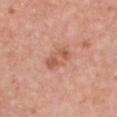Impression: The lesion was tiled from a total-body skin photograph and was not biopsied. Image and clinical context: The recorded lesion diameter is about 3.5 mm. The patient is a female aged 38–42. A roughly 15 mm field-of-view crop from a total-body skin photograph. Automated tile analysis of the lesion measured a footprint of about 6 mm², an eccentricity of roughly 0.85, and a symmetry-axis asymmetry near 0.2. The analysis additionally found a lesion color around L≈57 a*≈25 b*≈31 in CIELAB, roughly 9 lightness units darker than nearby skin, and a normalized border contrast of about 6.5. It also reported border irregularity of about 2.5 on a 0–10 scale and radial color variation of about 1. It also reported a nevus-likeness score of about 15/100 and lesion-presence confidence of about 100/100. Located on the chest.